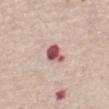Clinical impression: Captured during whole-body skin photography for melanoma surveillance; the lesion was not biopsied. Context: This is a white-light tile. The lesion is on the abdomen. Approximately 3 mm at its widest. A lesion tile, about 15 mm wide, cut from a 3D total-body photograph. A female subject, roughly 70 years of age. An algorithmic analysis of the crop reported a footprint of about 5 mm² and an outline eccentricity of about 0.75 (0 = round, 1 = elongated). And it measured a border-irregularity rating of about 3/10 and a color-variation rating of about 5.5/10. The analysis additionally found a nevus-likeness score of about 0/100 and lesion-presence confidence of about 100/100.